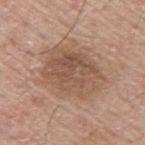Notes:
• biopsy status · catalogued during a skin exam; not biopsied
• location · the back
• acquisition · ~15 mm crop, total-body skin-cancer survey
• patient · male, about 55 years old
• tile lighting · white-light